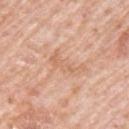Clinical impression:
The lesion was tiled from a total-body skin photograph and was not biopsied.
Context:
A male patient aged 78–82. The lesion is on the left upper arm. The lesion's longest dimension is about 4.5 mm. A region of skin cropped from a whole-body photographic capture, roughly 15 mm wide. Automated image analysis of the tile measured border irregularity of about 8 on a 0–10 scale, internal color variation of about 1 on a 0–10 scale, and a peripheral color-asymmetry measure near 0. The tile uses white-light illumination.